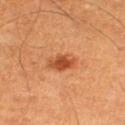{
  "site": "right thigh",
  "lighting": "cross-polarized",
  "patient": {
    "sex": "male",
    "age_approx": 60
  },
  "lesion_size": {
    "long_diameter_mm_approx": 3.0
  },
  "image": {
    "source": "total-body photography crop",
    "field_of_view_mm": 15
  }
}